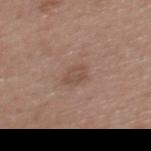Captured during whole-body skin photography for melanoma surveillance; the lesion was not biopsied.